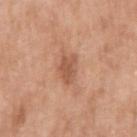This lesion was catalogued during total-body skin photography and was not selected for biopsy.
A male subject, roughly 65 years of age.
A 15 mm close-up extracted from a 3D total-body photography capture.
The lesion is located on the arm.